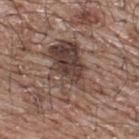| field | value |
|---|---|
| notes | imaged on a skin check; not biopsied |
| location | the upper back |
| acquisition | ~15 mm tile from a whole-body skin photo |
| automated metrics | a border-irregularity rating of about 7/10 and internal color variation of about 8.5 on a 0–10 scale |
| illumination | white-light illumination |
| lesion diameter | ~7.5 mm (longest diameter) |
| subject | male, aged around 65 |